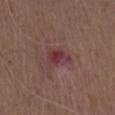No biopsy was performed on this lesion — it was imaged during a full skin examination and was not determined to be concerning. Imaged with white-light lighting. The lesion is on the chest. A male patient, about 70 years old. Automated image analysis of the tile measured a classifier nevus-likeness of about 0/100 and lesion-presence confidence of about 100/100. A region of skin cropped from a whole-body photographic capture, roughly 15 mm wide.